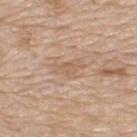This lesion was catalogued during total-body skin photography and was not selected for biopsy. A male subject about 80 years old. Located on the upper back. A 15 mm crop from a total-body photograph taken for skin-cancer surveillance.